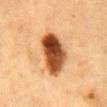| feature | finding |
|---|---|
| follow-up | no biopsy performed (imaged during a skin exam) |